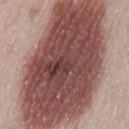Recorded during total-body skin imaging; not selected for excision or biopsy. The lesion is located on the back. The subject is a female aged 48–52. The lesion-visualizer software estimated a footprint of about 130 mm², an outline eccentricity of about 0.85 (0 = round, 1 = elongated), and a symmetry-axis asymmetry near 0.15. The analysis additionally found an average lesion color of about L≈48 a*≈22 b*≈22 (CIELAB), about 20 CIELAB-L* units darker than the surrounding skin, and a normalized lesion–skin contrast near 13. It also reported a nevus-likeness score of about 100/100 and a detector confidence of about 100 out of 100 that the crop contains a lesion. A 15 mm crop from a total-body photograph taken for skin-cancer surveillance. Captured under white-light illumination.